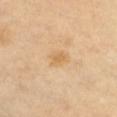Clinical impression: Captured during whole-body skin photography for melanoma surveillance; the lesion was not biopsied. Acquisition and patient details: The lesion is on the chest. This image is a 15 mm lesion crop taken from a total-body photograph. The patient is a female roughly 60 years of age. Captured under cross-polarized illumination.